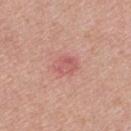notes — total-body-photography surveillance lesion; no biopsy
tile lighting — white-light illumination
image source — total-body-photography crop, ~15 mm field of view
patient — male, aged 43 to 47
size — about 3 mm
location — the right upper arm
automated metrics — a lesion area of about 5.5 mm², an eccentricity of roughly 0.65, and two-axis asymmetry of about 0.15; a border-irregularity index near 1/10 and a peripheral color-asymmetry measure near 1; a classifier nevus-likeness of about 0/100 and a lesion-detection confidence of about 100/100